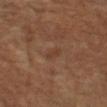Recorded during total-body skin imaging; not selected for excision or biopsy.
The lesion is located on the left forearm.
A male subject, aged 58 to 62.
An algorithmic analysis of the crop reported a border-irregularity index near 2.5/10 and radial color variation of about 0. The software also gave an automated nevus-likeness rating near 0 out of 100.
Captured under cross-polarized illumination.
Cropped from a total-body skin-imaging series; the visible field is about 15 mm.
The recorded lesion diameter is about 2.5 mm.